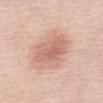No biopsy was performed on this lesion — it was imaged during a full skin examination and was not determined to be concerning. Cropped from a whole-body photographic skin survey; the tile spans about 15 mm. The lesion is on the front of the torso. Captured under white-light illumination. About 5 mm across. A female subject, in their 70s. Automated image analysis of the tile measured a footprint of about 16 mm² and two-axis asymmetry of about 0.2. The software also gave a mean CIELAB color near L≈64 a*≈23 b*≈28, a lesion–skin lightness drop of about 10, and a normalized border contrast of about 6. It also reported internal color variation of about 3.5 on a 0–10 scale and peripheral color asymmetry of about 1.